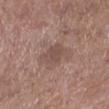Assessment:
Recorded during total-body skin imaging; not selected for excision or biopsy.
Acquisition and patient details:
Longest diameter approximately 2.5 mm. A roughly 15 mm field-of-view crop from a total-body skin photograph. From the leg. Captured under white-light illumination. A female subject approximately 50 years of age. The lesion-visualizer software estimated an outline eccentricity of about 0.45 (0 = round, 1 = elongated). It also reported a lesion-detection confidence of about 100/100.